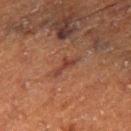Clinical impression:
Recorded during total-body skin imaging; not selected for excision or biopsy.
Acquisition and patient details:
From the right thigh. The lesion-visualizer software estimated border irregularity of about 7 on a 0–10 scale. The software also gave a classifier nevus-likeness of about 0/100 and a detector confidence of about 70 out of 100 that the crop contains a lesion. Cropped from a total-body skin-imaging series; the visible field is about 15 mm. A male subject, aged 73 to 77. Imaged with cross-polarized lighting. The lesion's longest dimension is about 3.5 mm.